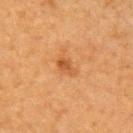Impression: This lesion was catalogued during total-body skin photography and was not selected for biopsy. Acquisition and patient details: Automated tile analysis of the lesion measured a footprint of about 3.5 mm², a shape eccentricity near 0.8, and a shape-asymmetry score of about 0.25 (0 = symmetric). It also reported a border-irregularity rating of about 2.5/10 and a peripheral color-asymmetry measure near 1. On the right upper arm. A region of skin cropped from a whole-body photographic capture, roughly 15 mm wide. The lesion's longest dimension is about 2.5 mm. A male patient about 85 years old or older.